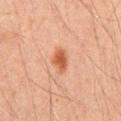Part of a total-body skin-imaging series; this lesion was reviewed on a skin check and was not flagged for biopsy. A 15 mm close-up tile from a total-body photography series done for melanoma screening. A male patient roughly 45 years of age. The lesion is on the chest. Approximately 3 mm at its widest. This is a cross-polarized tile. Automated tile analysis of the lesion measured a mean CIELAB color near L≈48 a*≈24 b*≈31, a lesion–skin lightness drop of about 10, and a normalized lesion–skin contrast near 8. The analysis additionally found border irregularity of about 2.5 on a 0–10 scale, a color-variation rating of about 2/10, and radial color variation of about 0.5.